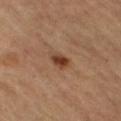biopsy status = catalogued during a skin exam; not biopsied
automated metrics = a footprint of about 3.5 mm², a shape eccentricity near 0.85, and two-axis asymmetry of about 0.2; a border-irregularity index near 2/10 and internal color variation of about 3.5 on a 0–10 scale; an automated nevus-likeness rating near 95 out of 100 and a detector confidence of about 100 out of 100 that the crop contains a lesion
body site = the right thigh
illumination = cross-polarized
diameter = ~2.5 mm (longest diameter)
patient = female, in their 70s
image = ~15 mm tile from a whole-body skin photo